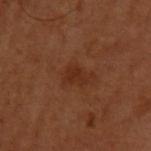Assessment: The lesion was photographed on a routine skin check and not biopsied; there is no pathology result. Clinical summary: From the arm. A male subject, roughly 50 years of age. This image is a 15 mm lesion crop taken from a total-body photograph.